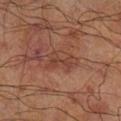| key | value |
|---|---|
| notes | no biopsy performed (imaged during a skin exam) |
| image-analysis metrics | an average lesion color of about L≈33 a*≈19 b*≈23 (CIELAB) |
| imaging modality | 15 mm crop, total-body photography |
| location | the leg |
| illumination | cross-polarized illumination |
| size | about 3.5 mm |
| subject | male, in their 70s |